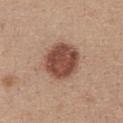On the chest. Captured under white-light illumination. About 4.5 mm across. Cropped from a total-body skin-imaging series; the visible field is about 15 mm. A female patient aged around 35. An algorithmic analysis of the crop reported a lesion area of about 16 mm² and a shape-asymmetry score of about 0.1 (0 = symmetric). The software also gave border irregularity of about 1 on a 0–10 scale, internal color variation of about 4 on a 0–10 scale, and radial color variation of about 1.5.